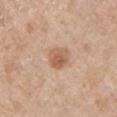Impression:
The lesion was tiled from a total-body skin photograph and was not biopsied.
Acquisition and patient details:
Captured under white-light illumination. A 15 mm crop from a total-body photograph taken for skin-cancer surveillance. The lesion is located on the left upper arm. The patient is a male aged approximately 65.